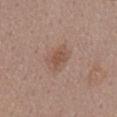Assessment: Captured during whole-body skin photography for melanoma surveillance; the lesion was not biopsied. Background: From the chest. Cropped from a whole-body photographic skin survey; the tile spans about 15 mm. The patient is a male in their mid-50s.